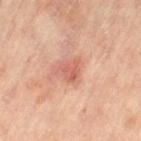workup: total-body-photography surveillance lesion; no biopsy
location: the right leg
tile lighting: cross-polarized
subject: female, in their mid-60s
acquisition: total-body-photography crop, ~15 mm field of view
lesion diameter: about 2.5 mm